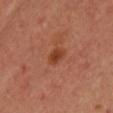Part of a total-body skin-imaging series; this lesion was reviewed on a skin check and was not flagged for biopsy.
The lesion is on the chest.
This is a cross-polarized tile.
Automated image analysis of the tile measured an outline eccentricity of about 0.75 (0 = round, 1 = elongated) and a symmetry-axis asymmetry near 0.2. And it measured roughly 8 lightness units darker than nearby skin and a normalized border contrast of about 7.5.
A region of skin cropped from a whole-body photographic capture, roughly 15 mm wide.
The subject is a female roughly 40 years of age.
About 2.5 mm across.